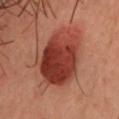Q: Is there a histopathology result?
A: no biopsy performed (imaged during a skin exam)
Q: Automated lesion metrics?
A: an area of roughly 27 mm² and a shape eccentricity near 0.8; a mean CIELAB color near L≈30 a*≈25 b*≈24, a lesion–skin lightness drop of about 12, and a lesion-to-skin contrast of about 11.5 (normalized; higher = more distinct); a border-irregularity index near 2/10, a within-lesion color-variation index near 5.5/10, and radial color variation of about 2; an automated nevus-likeness rating near 100 out of 100 and a lesion-detection confidence of about 100/100
Q: Lesion location?
A: the front of the torso
Q: Who is the patient?
A: male, aged around 40
Q: What is the imaging modality?
A: ~15 mm crop, total-body skin-cancer survey
Q: How large is the lesion?
A: about 7.5 mm